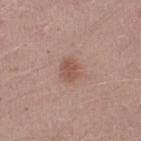patient = male, in their mid-20s
acquisition = ~15 mm tile from a whole-body skin photo
anatomic site = the arm
lesion diameter = ~2.5 mm (longest diameter)
illumination = white-light illumination